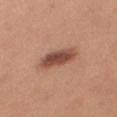Clinical impression:
The lesion was tiled from a total-body skin photograph and was not biopsied.
Image and clinical context:
Cropped from a total-body skin-imaging series; the visible field is about 15 mm. The subject is a female in their mid-20s. On the right thigh. Captured under white-light illumination. Longest diameter approximately 5 mm. The total-body-photography lesion software estimated a lesion area of about 9.5 mm², a shape eccentricity near 0.9, and a shape-asymmetry score of about 0.15 (0 = symmetric). It also reported an average lesion color of about L≈49 a*≈23 b*≈28 (CIELAB), roughly 14 lightness units darker than nearby skin, and a normalized lesion–skin contrast near 10.